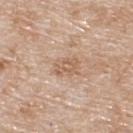Findings:
– biopsy status · no biopsy performed (imaged during a skin exam)
– site · the upper back
– image · 15 mm crop, total-body photography
– patient · male, about 80 years old